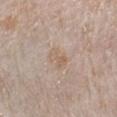This lesion was catalogued during total-body skin photography and was not selected for biopsy.
The patient is a female in their mid-60s.
The lesion is located on the left forearm.
A lesion tile, about 15 mm wide, cut from a 3D total-body photograph.